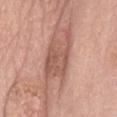Assessment: This lesion was catalogued during total-body skin photography and was not selected for biopsy. Clinical summary: About 7 mm across. The total-body-photography lesion software estimated a footprint of about 16 mm², an eccentricity of roughly 0.85, and a symmetry-axis asymmetry near 0.3. And it measured an average lesion color of about L≈56 a*≈23 b*≈27 (CIELAB), a lesion–skin lightness drop of about 11, and a normalized lesion–skin contrast near 7.5. And it measured a border-irregularity index near 5/10, a color-variation rating of about 3.5/10, and peripheral color asymmetry of about 1.5. This is a white-light tile. The patient is a female approximately 60 years of age. From the abdomen. A roughly 15 mm field-of-view crop from a total-body skin photograph.